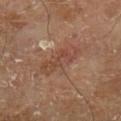This is a cross-polarized tile. The lesion's longest dimension is about 5 mm. The patient is a male in their 70s. A region of skin cropped from a whole-body photographic capture, roughly 15 mm wide. The lesion is located on the left lower leg.